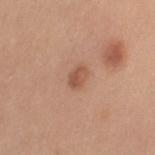Impression: Part of a total-body skin-imaging series; this lesion was reviewed on a skin check and was not flagged for biopsy. Clinical summary: A female patient, aged approximately 30. Measured at roughly 2.5 mm in maximum diameter. The lesion-visualizer software estimated an outline eccentricity of about 0.8 (0 = round, 1 = elongated) and a symmetry-axis asymmetry near 0.15. It also reported an average lesion color of about L≈54 a*≈23 b*≈31 (CIELAB) and a normalized lesion–skin contrast near 6.5. The software also gave a nevus-likeness score of about 70/100 and lesion-presence confidence of about 100/100. The tile uses white-light illumination. Cropped from a total-body skin-imaging series; the visible field is about 15 mm. The lesion is on the arm.